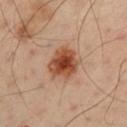Q: Was a biopsy performed?
A: total-body-photography surveillance lesion; no biopsy
Q: What did automated image analysis measure?
A: a lesion area of about 13 mm², an eccentricity of roughly 0.5, and a symmetry-axis asymmetry near 0.2; a border-irregularity rating of about 2.5/10, a within-lesion color-variation index near 6/10, and peripheral color asymmetry of about 1.5; a nevus-likeness score of about 100/100 and lesion-presence confidence of about 100/100
Q: What is the lesion's diameter?
A: ~4.5 mm (longest diameter)
Q: Who is the patient?
A: male, roughly 50 years of age
Q: Where on the body is the lesion?
A: the left upper arm
Q: What lighting was used for the tile?
A: cross-polarized illumination
Q: What is the imaging modality?
A: 15 mm crop, total-body photography